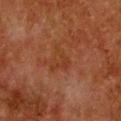| feature | finding |
|---|---|
| biopsy status | imaged on a skin check; not biopsied |
| acquisition | total-body-photography crop, ~15 mm field of view |
| subject | female, approximately 50 years of age |
| lesion diameter | about 3 mm |
| image-analysis metrics | a border-irregularity index near 9.5/10 and a peripheral color-asymmetry measure near 0; lesion-presence confidence of about 100/100 |
| anatomic site | the chest |
| illumination | cross-polarized |